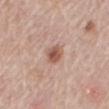subject — female, aged approximately 65
diameter — about 2.5 mm
acquisition — ~15 mm tile from a whole-body skin photo
anatomic site — the mid back
automated metrics — an outline eccentricity of about 0.6 (0 = round, 1 = elongated); a mean CIELAB color near L≈55 a*≈21 b*≈27 and about 12 CIELAB-L* units darker than the surrounding skin; an automated nevus-likeness rating near 80 out of 100 and a detector confidence of about 100 out of 100 that the crop contains a lesion
illumination — white-light illumination